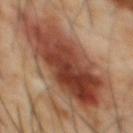Q: Was a biopsy performed?
A: imaged on a skin check; not biopsied
Q: How was the tile lit?
A: cross-polarized
Q: Patient demographics?
A: male, about 65 years old
Q: What is the imaging modality?
A: ~15 mm crop, total-body skin-cancer survey
Q: How large is the lesion?
A: about 12.5 mm
Q: What is the anatomic site?
A: the abdomen
Q: What did automated image analysis measure?
A: a lesion-to-skin contrast of about 10.5 (normalized; higher = more distinct); a border-irregularity index near 5.5/10 and peripheral color asymmetry of about 3